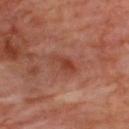{
  "biopsy_status": "not biopsied; imaged during a skin examination",
  "image": {
    "source": "total-body photography crop",
    "field_of_view_mm": 15
  },
  "automated_metrics": {
    "area_mm2_approx": 5.0,
    "eccentricity": 0.9,
    "shape_asymmetry": 0.25
  },
  "site": "upper back",
  "patient": {
    "sex": "male",
    "age_approx": 65
  }
}